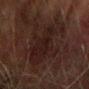<lesion>
  <image>
    <source>total-body photography crop</source>
    <field_of_view_mm>15</field_of_view_mm>
  </image>
  <patient>
    <sex>male</sex>
    <age_approx>75</age_approx>
  </patient>
  <site>right forearm</site>
</lesion>